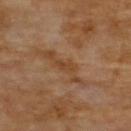Impression: Part of a total-body skin-imaging series; this lesion was reviewed on a skin check and was not flagged for biopsy. Clinical summary: Cropped from a total-body skin-imaging series; the visible field is about 15 mm. Approximately 2.5 mm at its widest. The tile uses cross-polarized illumination. From the upper back. A male subject in their 70s.